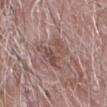From the left forearm. About 4.5 mm across. A 15 mm close-up extracted from a 3D total-body photography capture. A male subject roughly 75 years of age.